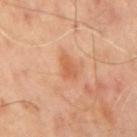Assessment:
Imaged during a routine full-body skin examination; the lesion was not biopsied and no histopathology is available.
Context:
Automated tile analysis of the lesion measured a lesion area of about 4 mm². The software also gave a lesion color around L≈57 a*≈26 b*≈36 in CIELAB, roughly 8 lightness units darker than nearby skin, and a lesion-to-skin contrast of about 6.5 (normalized; higher = more distinct). The subject is a male about 65 years old. The lesion's longest dimension is about 2.5 mm. Imaged with cross-polarized lighting. The lesion is located on the mid back. Cropped from a total-body skin-imaging series; the visible field is about 15 mm.